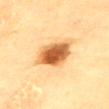Assessment: The lesion was tiled from a total-body skin photograph and was not biopsied. Image and clinical context: On the mid back. A 15 mm crop from a total-body photograph taken for skin-cancer surveillance. The patient is a female roughly 80 years of age. Imaged with cross-polarized lighting. Longest diameter approximately 6.5 mm.